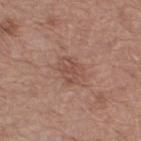<record>
  <biopsy_status>not biopsied; imaged during a skin examination</biopsy_status>
  <patient>
    <sex>female</sex>
    <age_approx>55</age_approx>
  </patient>
  <image>
    <source>total-body photography crop</source>
    <field_of_view_mm>15</field_of_view_mm>
  </image>
  <site>right thigh</site>
  <lesion_size>
    <long_diameter_mm_approx>3.5</long_diameter_mm_approx>
  </lesion_size>
  <lighting>white-light</lighting>
</record>